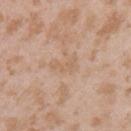The patient is a female in their mid-20s.
A 15 mm close-up extracted from a 3D total-body photography capture.
From the arm.